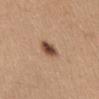The lesion was photographed on a routine skin check and not biopsied; there is no pathology result.
From the abdomen.
An algorithmic analysis of the crop reported an average lesion color of about L≈48 a*≈19 b*≈29 (CIELAB), a lesion–skin lightness drop of about 16, and a normalized lesion–skin contrast near 11. The analysis additionally found a classifier nevus-likeness of about 95/100.
A close-up tile cropped from a whole-body skin photograph, about 15 mm across.
Measured at roughly 2.5 mm in maximum diameter.
The subject is a female aged 33 to 37.